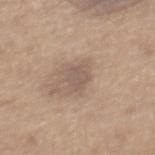Q: Was this lesion biopsied?
A: total-body-photography surveillance lesion; no biopsy
Q: Who is the patient?
A: male, aged 63 to 67
Q: What kind of image is this?
A: 15 mm crop, total-body photography
Q: Where on the body is the lesion?
A: the mid back